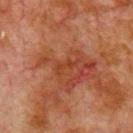biopsy status: imaged on a skin check; not biopsied
size: ~3 mm (longest diameter)
automated metrics: a lesion area of about 2 mm², an eccentricity of roughly 0.95, and a shape-asymmetry score of about 0.4 (0 = symmetric); a nevus-likeness score of about 0/100 and a detector confidence of about 95 out of 100 that the crop contains a lesion
lighting: cross-polarized illumination
body site: the chest
image: ~15 mm crop, total-body skin-cancer survey
subject: male, roughly 80 years of age